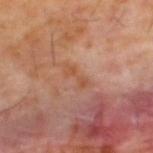workup: total-body-photography surveillance lesion; no biopsy
site: the upper back
TBP lesion metrics: a shape eccentricity near 0.95; a border-irregularity rating of about 5.5/10 and radial color variation of about 0; an automated nevus-likeness rating near 0 out of 100 and a lesion-detection confidence of about 100/100
illumination: cross-polarized illumination
acquisition: ~15 mm crop, total-body skin-cancer survey
patient: male, roughly 60 years of age
lesion size: about 3.5 mm